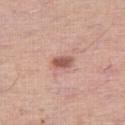Impression: Recorded during total-body skin imaging; not selected for excision or biopsy. Image and clinical context: The lesion is on the right thigh. Longest diameter approximately 2.5 mm. A 15 mm crop from a total-body photograph taken for skin-cancer surveillance. A female subject roughly 50 years of age. The lesion-visualizer software estimated a mean CIELAB color near L≈56 a*≈24 b*≈26, roughly 13 lightness units darker than nearby skin, and a normalized border contrast of about 8.5. It also reported border irregularity of about 1.5 on a 0–10 scale, a color-variation rating of about 3/10, and a peripheral color-asymmetry measure near 1. And it measured a nevus-likeness score of about 80/100 and a lesion-detection confidence of about 100/100. Captured under white-light illumination.